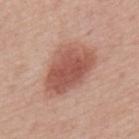Assessment: No biopsy was performed on this lesion — it was imaged during a full skin examination and was not determined to be concerning. Clinical summary: Measured at roughly 7.5 mm in maximum diameter. Imaged with white-light lighting. The lesion is on the mid back. A lesion tile, about 15 mm wide, cut from a 3D total-body photograph. Automated image analysis of the tile measured an outline eccentricity of about 0.8 (0 = round, 1 = elongated) and a shape-asymmetry score of about 0.25 (0 = symmetric). And it measured an average lesion color of about L≈54 a*≈25 b*≈27 (CIELAB) and a lesion–skin lightness drop of about 12. And it measured a border-irregularity index near 2.5/10, internal color variation of about 4.5 on a 0–10 scale, and a peripheral color-asymmetry measure near 1.5. It also reported a nevus-likeness score of about 100/100 and a detector confidence of about 100 out of 100 that the crop contains a lesion. A male subject, approximately 80 years of age.